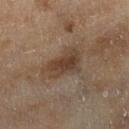Clinical impression: Captured during whole-body skin photography for melanoma surveillance; the lesion was not biopsied. Background: A 15 mm close-up extracted from a 3D total-body photography capture. The patient is a female aged 58–62. The recorded lesion diameter is about 5 mm. An algorithmic analysis of the crop reported a lesion color around L≈36 a*≈14 b*≈24 in CIELAB, about 9 CIELAB-L* units darker than the surrounding skin, and a normalized border contrast of about 8. The software also gave a border-irregularity index near 3.5/10 and a peripheral color-asymmetry measure near 1. This is a cross-polarized tile. The lesion is located on the right lower leg.